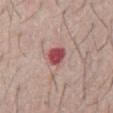The lesion's longest dimension is about 2.5 mm. An algorithmic analysis of the crop reported an average lesion color of about L≈49 a*≈32 b*≈20 (CIELAB), a lesion–skin lightness drop of about 16, and a normalized border contrast of about 11. And it measured a detector confidence of about 100 out of 100 that the crop contains a lesion. The patient is a male approximately 65 years of age. Cropped from a whole-body photographic skin survey; the tile spans about 15 mm. Imaged with white-light lighting. On the abdomen.